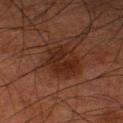Q: Was a biopsy performed?
A: total-body-photography surveillance lesion; no biopsy
Q: What is the imaging modality?
A: ~15 mm crop, total-body skin-cancer survey
Q: Lesion size?
A: ≈4 mm
Q: What are the patient's age and sex?
A: male, aged 78–82
Q: What lighting was used for the tile?
A: cross-polarized illumination
Q: What is the anatomic site?
A: the left lower leg